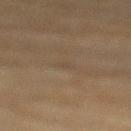Assessment:
This lesion was catalogued during total-body skin photography and was not selected for biopsy.
Clinical summary:
Imaged with cross-polarized lighting. A female subject, aged 78 to 82. Measured at roughly 1.5 mm in maximum diameter. The lesion-visualizer software estimated a lesion area of about 1.5 mm², an outline eccentricity of about 0.75 (0 = round, 1 = elongated), and two-axis asymmetry of about 0.25. It also reported a mean CIELAB color near L≈38 a*≈11 b*≈24, about 3 CIELAB-L* units darker than the surrounding skin, and a lesion-to-skin contrast of about 3 (normalized; higher = more distinct). The analysis additionally found a border-irregularity rating of about 2/10, internal color variation of about 0 on a 0–10 scale, and a peripheral color-asymmetry measure near 0. Cropped from a total-body skin-imaging series; the visible field is about 15 mm. The lesion is on the mid back.